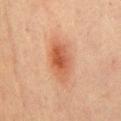image source: ~15 mm crop, total-body skin-cancer survey | image-analysis metrics: a mean CIELAB color near L≈47 a*≈23 b*≈31 and a lesion-to-skin contrast of about 8 (normalized; higher = more distinct) | site: the mid back | lesion diameter: ≈5.5 mm | subject: male, in their 70s | illumination: cross-polarized illumination.